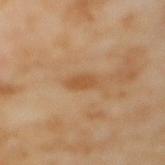Q: Was this lesion biopsied?
A: catalogued during a skin exam; not biopsied
Q: Lesion location?
A: the mid back
Q: How was the tile lit?
A: cross-polarized illumination
Q: How was this image acquired?
A: ~15 mm tile from a whole-body skin photo
Q: Who is the patient?
A: female, in their mid- to late 50s
Q: What is the lesion's diameter?
A: about 3 mm